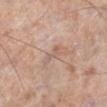Assessment: The lesion was tiled from a total-body skin photograph and was not biopsied. Acquisition and patient details: A region of skin cropped from a whole-body photographic capture, roughly 15 mm wide. Located on the left lower leg. The recorded lesion diameter is about 2.5 mm. Automated image analysis of the tile measured an area of roughly 2 mm², an eccentricity of roughly 0.95, and a symmetry-axis asymmetry near 0.45. The software also gave a lesion color around L≈59 a*≈18 b*≈26 in CIELAB and a lesion-to-skin contrast of about 5 (normalized; higher = more distinct). It also reported border irregularity of about 5.5 on a 0–10 scale, internal color variation of about 0 on a 0–10 scale, and radial color variation of about 0. The analysis additionally found an automated nevus-likeness rating near 0 out of 100 and lesion-presence confidence of about 75/100. A male subject roughly 75 years of age. This is a white-light tile.